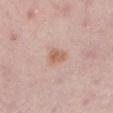Recorded during total-body skin imaging; not selected for excision or biopsy. The patient is a female approximately 45 years of age. Captured under white-light illumination. About 2.5 mm across. The total-body-photography lesion software estimated a detector confidence of about 100 out of 100 that the crop contains a lesion. Cropped from a total-body skin-imaging series; the visible field is about 15 mm. From the left lower leg.